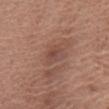lesion diameter: ≈3 mm | imaging modality: total-body-photography crop, ~15 mm field of view | automated metrics: a nevus-likeness score of about 0/100 and lesion-presence confidence of about 100/100 | tile lighting: white-light illumination | patient: female, about 80 years old | body site: the chest.